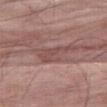This lesion was catalogued during total-body skin photography and was not selected for biopsy. Approximately 4.5 mm at its widest. Imaged with white-light lighting. A lesion tile, about 15 mm wide, cut from a 3D total-body photograph. The lesion is on the right thigh. The subject is a male roughly 80 years of age.